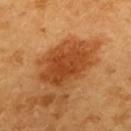Captured during whole-body skin photography for melanoma surveillance; the lesion was not biopsied. On the back. A female subject about 55 years old. A 15 mm close-up tile from a total-body photography series done for melanoma screening.